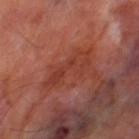{"biopsy_status": "not biopsied; imaged during a skin examination", "site": "leg", "image": {"source": "total-body photography crop", "field_of_view_mm": 15}, "patient": {"sex": "male", "age_approx": 70}, "automated_metrics": {"area_mm2_approx": 8.5, "shape_asymmetry": 0.45, "border_irregularity_0_10": 6.5, "peripheral_color_asymmetry": 1.0}, "lighting": "cross-polarized"}